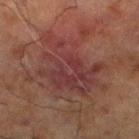Recorded during total-body skin imaging; not selected for excision or biopsy.
The subject is a male in their 70s.
The tile uses cross-polarized illumination.
The lesion is located on the left lower leg.
A 15 mm close-up extracted from a 3D total-body photography capture.
The recorded lesion diameter is about 9.5 mm.